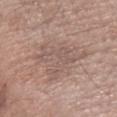  biopsy_status: not biopsied; imaged during a skin examination
  site: left forearm
  lighting: white-light
  lesion_size:
    long_diameter_mm_approx: 6.5
  image:
    source: total-body photography crop
    field_of_view_mm: 15
  patient:
    sex: male
    age_approx: 55